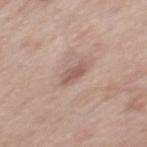notes: no biopsy performed (imaged during a skin exam) | location: the mid back | imaging modality: ~15 mm crop, total-body skin-cancer survey | patient: male, roughly 55 years of age.